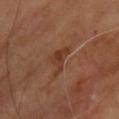Impression: This lesion was catalogued during total-body skin photography and was not selected for biopsy. Background: A 15 mm close-up tile from a total-body photography series done for melanoma screening. A male patient, aged 63 to 67. An algorithmic analysis of the crop reported an area of roughly 5.5 mm², an eccentricity of roughly 0.7, and a shape-asymmetry score of about 0.6 (0 = symmetric). It also reported internal color variation of about 2.5 on a 0–10 scale and radial color variation of about 1. And it measured a classifier nevus-likeness of about 0/100. From the chest. About 3.5 mm across. This is a cross-polarized tile.